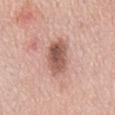Q: Was a biopsy performed?
A: catalogued during a skin exam; not biopsied
Q: What are the patient's age and sex?
A: male, in their mid- to late 50s
Q: Where on the body is the lesion?
A: the mid back
Q: What kind of image is this?
A: ~15 mm tile from a whole-body skin photo
Q: What lighting was used for the tile?
A: white-light
Q: How large is the lesion?
A: ≈5 mm